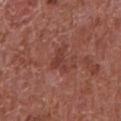Part of a total-body skin-imaging series; this lesion was reviewed on a skin check and was not flagged for biopsy.
From the front of the torso.
A male subject, roughly 65 years of age.
A region of skin cropped from a whole-body photographic capture, roughly 15 mm wide.